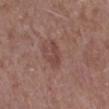The lesion was tiled from a total-body skin photograph and was not biopsied.